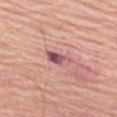Q: Is there a histopathology result?
A: total-body-photography surveillance lesion; no biopsy
Q: Who is the patient?
A: male, in their 80s
Q: What did automated image analysis measure?
A: an average lesion color of about L≈55 a*≈27 b*≈19 (CIELAB), a lesion–skin lightness drop of about 13, and a normalized border contrast of about 9.5; a classifier nevus-likeness of about 0/100
Q: What kind of image is this?
A: ~15 mm tile from a whole-body skin photo
Q: Lesion location?
A: the left thigh
Q: What is the lesion's diameter?
A: ~3.5 mm (longest diameter)